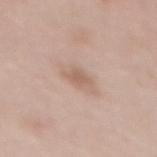workup — catalogued during a skin exam; not biopsied | patient — female, aged approximately 50 | imaging modality — ~15 mm tile from a whole-body skin photo | location — the mid back | size — ~2.5 mm (longest diameter).